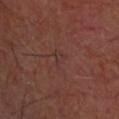Clinical impression:
The lesion was photographed on a routine skin check and not biopsied; there is no pathology result.
Clinical summary:
The tile uses cross-polarized illumination. An algorithmic analysis of the crop reported a symmetry-axis asymmetry near 0.55. The analysis additionally found a lesion color around L≈31 a*≈19 b*≈18 in CIELAB, roughly 4 lightness units darker than nearby skin, and a normalized border contrast of about 4.5. It also reported border irregularity of about 5.5 on a 0–10 scale, a color-variation rating of about 1/10, and a peripheral color-asymmetry measure near 0. And it measured a nevus-likeness score of about 0/100 and a detector confidence of about 60 out of 100 that the crop contains a lesion. A 15 mm close-up tile from a total-body photography series done for melanoma screening. Measured at roughly 2.5 mm in maximum diameter. A male patient, approximately 60 years of age. The lesion is on the head or neck.